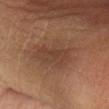Q: Was a biopsy performed?
A: no biopsy performed (imaged during a skin exam)